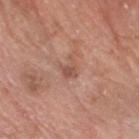imaging modality=15 mm crop, total-body photography
body site=the head or neck
lesion diameter=about 3 mm
TBP lesion metrics=a border-irregularity rating of about 6/10, internal color variation of about 1 on a 0–10 scale, and peripheral color asymmetry of about 0.5
tile lighting=white-light
subject=male, aged around 80